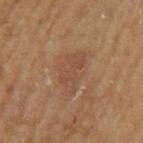biopsy status: imaged on a skin check; not biopsied | patient: male, in their mid-60s | location: the left upper arm | image source: 15 mm crop, total-body photography | size: ~5.5 mm (longest diameter) | lighting: cross-polarized illumination.